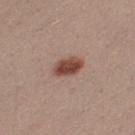Imaged during a routine full-body skin examination; the lesion was not biopsied and no histopathology is available.
A close-up tile cropped from a whole-body skin photograph, about 15 mm across.
A female patient about 25 years old.
Captured under white-light illumination.
From the left thigh.
Automated image analysis of the tile measured a lesion-detection confidence of about 100/100.